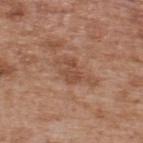| field | value |
|---|---|
| follow-up | total-body-photography surveillance lesion; no biopsy |
| patient | male, in their mid-60s |
| imaging modality | total-body-photography crop, ~15 mm field of view |
| lesion diameter | about 3 mm |
| image-analysis metrics | a shape eccentricity near 0.8 and a shape-asymmetry score of about 0.35 (0 = symmetric); an average lesion color of about L≈48 a*≈22 b*≈30 (CIELAB) and a lesion–skin lightness drop of about 8; a border-irregularity index near 4/10 and radial color variation of about 0.5 |
| illumination | white-light |
| anatomic site | the upper back |